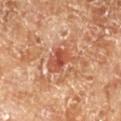biopsy status: total-body-photography surveillance lesion; no biopsy
subject: male, aged approximately 70
anatomic site: the left lower leg
acquisition: ~15 mm tile from a whole-body skin photo
image-analysis metrics: a lesion area of about 7.5 mm² and a symmetry-axis asymmetry near 0.3; a lesion–skin lightness drop of about 11 and a lesion-to-skin contrast of about 7.5 (normalized; higher = more distinct); a border-irregularity index near 4/10, a color-variation rating of about 4.5/10, and radial color variation of about 1.5
tile lighting: cross-polarized illumination